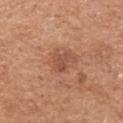– workup: total-body-photography surveillance lesion; no biopsy
– automated metrics: an eccentricity of roughly 0.7 and a symmetry-axis asymmetry near 0.35; a lesion color around L≈50 a*≈24 b*≈31 in CIELAB, a lesion–skin lightness drop of about 8, and a normalized lesion–skin contrast near 6; a border-irregularity rating of about 3.5/10 and radial color variation of about 0.5; an automated nevus-likeness rating near 5 out of 100
– illumination: white-light
– image: total-body-photography crop, ~15 mm field of view
– site: the left upper arm
– lesion size: ≈2.5 mm
– subject: female, approximately 55 years of age